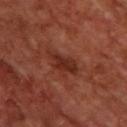Q: What is the anatomic site?
A: the upper back
Q: Who is the patient?
A: male, aged around 70
Q: What kind of image is this?
A: ~15 mm tile from a whole-body skin photo
Q: What is the lesion's diameter?
A: about 4.5 mm
Q: Illumination type?
A: cross-polarized